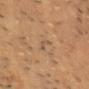Captured during whole-body skin photography for melanoma surveillance; the lesion was not biopsied.
The total-body-photography lesion software estimated a symmetry-axis asymmetry near 0.5.
The lesion's longest dimension is about 2.5 mm.
The lesion is located on the chest.
The tile uses cross-polarized illumination.
A close-up tile cropped from a whole-body skin photograph, about 15 mm across.
The patient is a male about 85 years old or older.